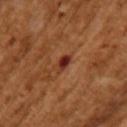body site = the left upper arm
tile lighting = cross-polarized
size = ≈2.5 mm
TBP lesion metrics = a border-irregularity index near 2.5/10, a within-lesion color-variation index near 6.5/10, and peripheral color asymmetry of about 2; a classifier nevus-likeness of about 0/100 and a detector confidence of about 100 out of 100 that the crop contains a lesion
patient = female, aged 53 to 57
acquisition = total-body-photography crop, ~15 mm field of view